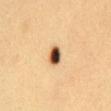The lesion was photographed on a routine skin check and not biopsied; there is no pathology result.
Longest diameter approximately 2.5 mm.
The lesion is on the chest.
A close-up tile cropped from a whole-body skin photograph, about 15 mm across.
The subject is a female aged 28–32.